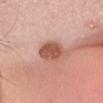{
  "site": "head or neck",
  "lesion_size": {
    "long_diameter_mm_approx": 3.5
  },
  "lighting": "white-light",
  "automated_metrics": {
    "area_mm2_approx": 8.5,
    "eccentricity": 0.55,
    "shape_asymmetry": 0.1,
    "border_irregularity_0_10": 1.5,
    "peripheral_color_asymmetry": 1.0,
    "nevus_likeness_0_100": 70,
    "lesion_detection_confidence_0_100": 100
  },
  "image": {
    "source": "total-body photography crop",
    "field_of_view_mm": 15
  },
  "patient": {
    "sex": "female",
    "age_approx": 25
  }
}